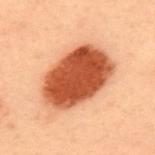Assessment:
Part of a total-body skin-imaging series; this lesion was reviewed on a skin check and was not flagged for biopsy.
Image and clinical context:
This is a cross-polarized tile. Automated tile analysis of the lesion measured a footprint of about 33 mm² and an eccentricity of roughly 0.8. It also reported internal color variation of about 4 on a 0–10 scale and a peripheral color-asymmetry measure near 1.5. A roughly 15 mm field-of-view crop from a total-body skin photograph. A male patient, aged around 55. The lesion is located on the upper back. About 8 mm across.